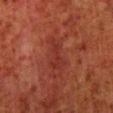Case summary:
* biopsy status · imaged on a skin check; not biopsied
* automated lesion analysis · a lesion color around L≈26 a*≈26 b*≈24 in CIELAB, about 5 CIELAB-L* units darker than the surrounding skin, and a normalized border contrast of about 5.5; a nevus-likeness score of about 0/100 and a detector confidence of about 95 out of 100 that the crop contains a lesion
* diameter · ≈5 mm
* imaging modality · ~15 mm crop, total-body skin-cancer survey
* site · the right lower leg
* subject · male, aged 78–82